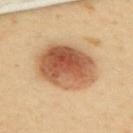Q: Is there a histopathology result?
A: imaged on a skin check; not biopsied
Q: Illumination type?
A: cross-polarized
Q: What is the lesion's diameter?
A: about 7 mm
Q: What did automated image analysis measure?
A: a nevus-likeness score of about 100/100
Q: How was this image acquired?
A: total-body-photography crop, ~15 mm field of view
Q: Lesion location?
A: the upper back
Q: Who is the patient?
A: female, aged 58 to 62